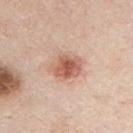  biopsy_status: not biopsied; imaged during a skin examination
  image:
    source: total-body photography crop
    field_of_view_mm: 15
  patient:
    sex: male
    age_approx: 45
  site: chest